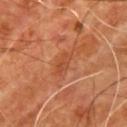• notes · imaged on a skin check; not biopsied
• tile lighting · cross-polarized
• patient · male, in their mid-50s
• automated metrics · a color-variation rating of about 2/10 and radial color variation of about 0.5
• image source · total-body-photography crop, ~15 mm field of view
• size · about 3 mm
• location · the chest